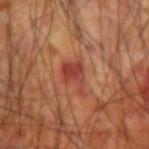{
  "biopsy_status": "not biopsied; imaged during a skin examination",
  "image": {
    "source": "total-body photography crop",
    "field_of_view_mm": 15
  },
  "site": "right upper arm",
  "lesion_size": {
    "long_diameter_mm_approx": 3.5
  },
  "patient": {
    "sex": "male",
    "age_approx": 60
  }
}